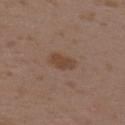Part of a total-body skin-imaging series; this lesion was reviewed on a skin check and was not flagged for biopsy. A female patient in their mid- to late 30s. From the upper back. This image is a 15 mm lesion crop taken from a total-body photograph.